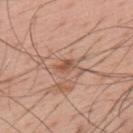Part of a total-body skin-imaging series; this lesion was reviewed on a skin check and was not flagged for biopsy.